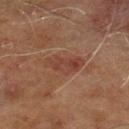Part of a total-body skin-imaging series; this lesion was reviewed on a skin check and was not flagged for biopsy.
The patient is a male aged 68–72.
A lesion tile, about 15 mm wide, cut from a 3D total-body photograph.
This is a cross-polarized tile.
About 4 mm across.
From the left lower leg.